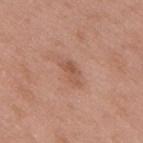Clinical impression: No biopsy was performed on this lesion — it was imaged during a full skin examination and was not determined to be concerning. Acquisition and patient details: The subject is a male aged 48–52. The tile uses white-light illumination. A roughly 15 mm field-of-view crop from a total-body skin photograph. Longest diameter approximately 3 mm. The lesion is on the upper back.